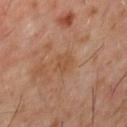A male patient, aged 58–62.
On the back.
This is a cross-polarized tile.
The lesion's longest dimension is about 2.5 mm.
A roughly 15 mm field-of-view crop from a total-body skin photograph.
The total-body-photography lesion software estimated an area of roughly 4.5 mm² and an outline eccentricity of about 0.4 (0 = round, 1 = elongated). It also reported roughly 5 lightness units darker than nearby skin and a normalized border contrast of about 5. It also reported border irregularity of about 3 on a 0–10 scale, internal color variation of about 2 on a 0–10 scale, and peripheral color asymmetry of about 0.5. The analysis additionally found a detector confidence of about 100 out of 100 that the crop contains a lesion.